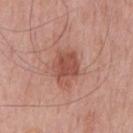| key | value |
|---|---|
| biopsy status | no biopsy performed (imaged during a skin exam) |
| subject | male, roughly 75 years of age |
| body site | the front of the torso |
| image | 15 mm crop, total-body photography |